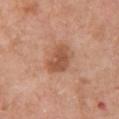{"image": {"source": "total-body photography crop", "field_of_view_mm": 15}, "patient": {"sex": "female", "age_approx": 60}, "automated_metrics": {"area_mm2_approx": 9.0, "eccentricity": 0.7, "shape_asymmetry": 0.25, "border_irregularity_0_10": 2.5, "color_variation_0_10": 3.0, "peripheral_color_asymmetry": 1.0, "nevus_likeness_0_100": 55, "lesion_detection_confidence_0_100": 100}, "lighting": "white-light", "lesion_size": {"long_diameter_mm_approx": 4.0}, "site": "chest"}